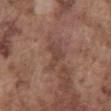  biopsy_status: not biopsied; imaged during a skin examination
  site: abdomen
  automated_metrics:
    area_mm2_approx: 4.5
    shape_asymmetry: 0.65
  patient:
    sex: male
    age_approx: 75
  lesion_size:
    long_diameter_mm_approx: 3.5
  image:
    source: total-body photography crop
    field_of_view_mm: 15
  lighting: white-light